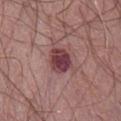{
  "biopsy_status": "not biopsied; imaged during a skin examination",
  "image": {
    "source": "total-body photography crop",
    "field_of_view_mm": 15
  },
  "site": "front of the torso",
  "patient": {
    "sex": "female",
    "age_approx": 65
  },
  "automated_metrics": {
    "border_irregularity_0_10": 2.0,
    "color_variation_0_10": 4.5,
    "peripheral_color_asymmetry": 1.5
  },
  "lesion_size": {
    "long_diameter_mm_approx": 3.0
  }
}